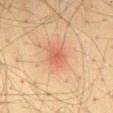Clinical impression: No biopsy was performed on this lesion — it was imaged during a full skin examination and was not determined to be concerning. Background: On the mid back. A male subject, aged 33 to 37. Automated tile analysis of the lesion measured a within-lesion color-variation index near 2.5/10 and peripheral color asymmetry of about 0.5. The analysis additionally found a classifier nevus-likeness of about 0/100 and a lesion-detection confidence of about 100/100. The recorded lesion diameter is about 2.5 mm. This image is a 15 mm lesion crop taken from a total-body photograph. Captured under cross-polarized illumination.